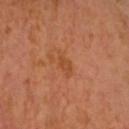{
  "biopsy_status": "not biopsied; imaged during a skin examination",
  "patient": {
    "sex": "male",
    "age_approx": 65
  },
  "automated_metrics": {
    "area_mm2_approx": 4.0,
    "shape_asymmetry": 0.3,
    "border_irregularity_0_10": 3.0,
    "color_variation_0_10": 1.5,
    "peripheral_color_asymmetry": 0.5
  },
  "lesion_size": {
    "long_diameter_mm_approx": 3.0
  },
  "image": {
    "source": "total-body photography crop",
    "field_of_view_mm": 15
  }
}